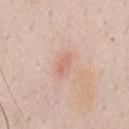Impression: Captured during whole-body skin photography for melanoma surveillance; the lesion was not biopsied. Acquisition and patient details: Cropped from a whole-body photographic skin survey; the tile spans about 15 mm. Located on the chest. A male subject about 50 years old.